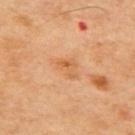{"biopsy_status": "not biopsied; imaged during a skin examination", "lighting": "cross-polarized", "patient": {"sex": "male", "age_approx": 45}, "image": {"source": "total-body photography crop", "field_of_view_mm": 15}, "site": "upper back"}